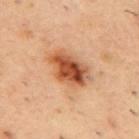This lesion was catalogued during total-body skin photography and was not selected for biopsy. Captured under cross-polarized illumination. Measured at roughly 5 mm in maximum diameter. The lesion is located on the upper back. This image is a 15 mm lesion crop taken from a total-body photograph. A male patient aged approximately 55.